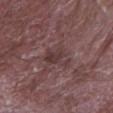Assessment: Imaged during a routine full-body skin examination; the lesion was not biopsied and no histopathology is available. Background: About 4.5 mm across. A male patient, aged around 65. A region of skin cropped from a whole-body photographic capture, roughly 15 mm wide. The lesion-visualizer software estimated a lesion color around L≈36 a*≈18 b*≈16 in CIELAB and about 7 CIELAB-L* units darker than the surrounding skin. And it measured a within-lesion color-variation index near 3/10 and a peripheral color-asymmetry measure near 1. Imaged with white-light lighting. On the arm.